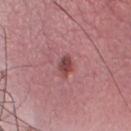No biopsy was performed on this lesion — it was imaged during a full skin examination and was not determined to be concerning.
From the chest.
Cropped from a whole-body photographic skin survey; the tile spans about 15 mm.
A male patient aged approximately 35.